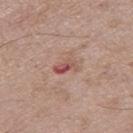A male subject, approximately 50 years of age.
Longest diameter approximately 3 mm.
A 15 mm close-up tile from a total-body photography series done for melanoma screening.
Imaged with white-light lighting.
The lesion is on the leg.